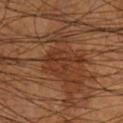* notes · imaged on a skin check; not biopsied
* body site · the right lower leg
* diameter · ~5 mm (longest diameter)
* subject · male, aged approximately 55
* image · 15 mm crop, total-body photography